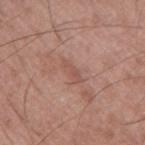Clinical impression: Captured during whole-body skin photography for melanoma surveillance; the lesion was not biopsied. Context: This is a white-light tile. Cropped from a total-body skin-imaging series; the visible field is about 15 mm. Measured at roughly 3.5 mm in maximum diameter. A male subject aged around 60. On the mid back.